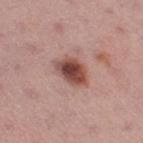Findings:
• automated metrics: border irregularity of about 2.5 on a 0–10 scale and internal color variation of about 6.5 on a 0–10 scale
• body site: the right thigh
• illumination: white-light
• lesion size: about 4 mm
• patient: female, about 35 years old
• imaging modality: total-body-photography crop, ~15 mm field of view